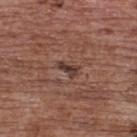biopsy status — no biopsy performed (imaged during a skin exam) | size — ~2.5 mm (longest diameter) | illumination — white-light illumination | anatomic site — the back | automated metrics — an area of roughly 3 mm², a shape eccentricity near 0.85, and a shape-asymmetry score of about 0.35 (0 = symmetric); a mean CIELAB color near L≈38 a*≈20 b*≈22 and a lesion-to-skin contrast of about 8.5 (normalized; higher = more distinct); a border-irregularity rating of about 4/10 and a within-lesion color-variation index near 0.5/10 | subject — female, roughly 65 years of age | acquisition — 15 mm crop, total-body photography.